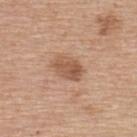Background: The subject is a male aged around 65. A region of skin cropped from a whole-body photographic capture, roughly 15 mm wide. From the upper back.